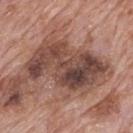Impression: The lesion was photographed on a routine skin check and not biopsied; there is no pathology result. Clinical summary: The lesion's longest dimension is about 9.5 mm. A close-up tile cropped from a whole-body skin photograph, about 15 mm across. The lesion is on the back. An algorithmic analysis of the crop reported a lesion color around L≈44 a*≈20 b*≈24 in CIELAB, roughly 14 lightness units darker than nearby skin, and a normalized border contrast of about 10.5. And it measured border irregularity of about 5 on a 0–10 scale and a color-variation rating of about 9/10. And it measured a nevus-likeness score of about 0/100. The subject is a male approximately 70 years of age.